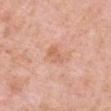No biopsy was performed on this lesion — it was imaged during a full skin examination and was not determined to be concerning. Automated image analysis of the tile measured a shape eccentricity near 0.8. And it measured a border-irregularity rating of about 2.5/10 and peripheral color asymmetry of about 0.5. The analysis additionally found a classifier nevus-likeness of about 0/100 and a lesion-detection confidence of about 100/100. Located on the front of the torso. A lesion tile, about 15 mm wide, cut from a 3D total-body photograph. The subject is a female about 40 years old.